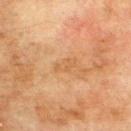Imaged during a routine full-body skin examination; the lesion was not biopsied and no histopathology is available. A 15 mm close-up tile from a total-body photography series done for melanoma screening. A male patient about 75 years old. Located on the upper back.